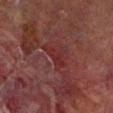Q: Is there a histopathology result?
A: no biopsy performed (imaged during a skin exam)
Q: What are the patient's age and sex?
A: male, in their 70s
Q: What lighting was used for the tile?
A: cross-polarized illumination
Q: Automated lesion metrics?
A: an area of roughly 6 mm², an outline eccentricity of about 0.7 (0 = round, 1 = elongated), and two-axis asymmetry of about 0.55; a border-irregularity rating of about 6.5/10, a within-lesion color-variation index near 2/10, and peripheral color asymmetry of about 0.5
Q: What is the lesion's diameter?
A: about 3.5 mm
Q: How was this image acquired?
A: ~15 mm tile from a whole-body skin photo
Q: What is the anatomic site?
A: the left lower leg